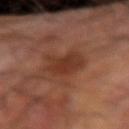illumination: cross-polarized illumination
patient: male, approximately 70 years of age
image source: 15 mm crop, total-body photography
diameter: ≈5 mm
location: the right forearm